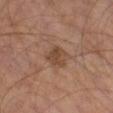{
  "biopsy_status": "not biopsied; imaged during a skin examination",
  "site": "right leg",
  "image": {
    "source": "total-body photography crop",
    "field_of_view_mm": 15
  },
  "lesion_size": {
    "long_diameter_mm_approx": 2.5
  },
  "automated_metrics": {
    "cielab_L": 42,
    "cielab_a": 18,
    "cielab_b": 28,
    "vs_skin_darker_L": 8.0,
    "border_irregularity_0_10": 2.5
  },
  "patient": {
    "sex": "male",
    "age_approx": 60
  },
  "lighting": "cross-polarized"
}